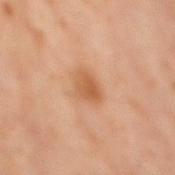- follow-up: imaged on a skin check; not biopsied
- patient: male, in their 60s
- diameter: ≈3.5 mm
- acquisition: ~15 mm tile from a whole-body skin photo
- TBP lesion metrics: an outline eccentricity of about 0.75 (0 = round, 1 = elongated) and a symmetry-axis asymmetry near 0.3; a mean CIELAB color near L≈54 a*≈22 b*≈35, roughly 9 lightness units darker than nearby skin, and a lesion-to-skin contrast of about 7 (normalized; higher = more distinct); a border-irregularity index near 2.5/10 and a within-lesion color-variation index near 3.5/10
- location: the mid back